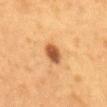notes: no biopsy performed (imaged during a skin exam) | patient: male, roughly 55 years of age | anatomic site: the back | imaging modality: ~15 mm tile from a whole-body skin photo | diameter: ≈3 mm.